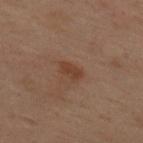Impression:
This lesion was catalogued during total-body skin photography and was not selected for biopsy.
Image and clinical context:
The lesion is located on the upper back. Cropped from a total-body skin-imaging series; the visible field is about 15 mm. Approximately 3 mm at its widest. A female patient in their mid- to late 50s. The total-body-photography lesion software estimated a lesion color around L≈32 a*≈17 b*≈25 in CIELAB and roughly 6 lightness units darker than nearby skin.